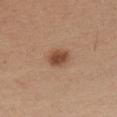Q: Is there a histopathology result?
A: total-body-photography surveillance lesion; no biopsy
Q: How was the tile lit?
A: white-light
Q: Patient demographics?
A: male, aged around 30
Q: What is the anatomic site?
A: the chest
Q: How large is the lesion?
A: ~3 mm (longest diameter)
Q: How was this image acquired?
A: 15 mm crop, total-body photography